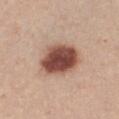{"biopsy_status": "not biopsied; imaged during a skin examination", "patient": {"sex": "female", "age_approx": 25}, "lighting": "white-light", "site": "front of the torso", "image": {"source": "total-body photography crop", "field_of_view_mm": 15}}